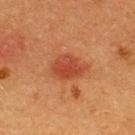No biopsy was performed on this lesion — it was imaged during a full skin examination and was not determined to be concerning.
This image is a 15 mm lesion crop taken from a total-body photograph.
Imaged with cross-polarized lighting.
The subject is a male approximately 40 years of age.
The lesion is located on the back.